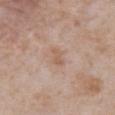On the abdomen. The subject is a male approximately 65 years of age. A 15 mm close-up extracted from a 3D total-body photography capture. The lesion's longest dimension is about 2.5 mm. Automated tile analysis of the lesion measured an area of roughly 3 mm², an outline eccentricity of about 0.85 (0 = round, 1 = elongated), and a symmetry-axis asymmetry near 0.35. And it measured about 7 CIELAB-L* units darker than the surrounding skin.